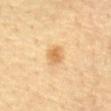{
  "biopsy_status": "not biopsied; imaged during a skin examination",
  "patient": {
    "sex": "female",
    "age_approx": 55
  },
  "image": {
    "source": "total-body photography crop",
    "field_of_view_mm": 15
  },
  "lesion_size": {
    "long_diameter_mm_approx": 2.5
  },
  "automated_metrics": {
    "area_mm2_approx": 5.0,
    "shape_asymmetry": 0.15,
    "cielab_L": 57,
    "cielab_a": 16,
    "cielab_b": 37,
    "vs_skin_darker_L": 9.0,
    "vs_skin_contrast_norm": 6.5
  },
  "site": "abdomen",
  "lighting": "cross-polarized"
}